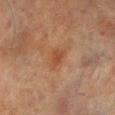Captured during whole-body skin photography for melanoma surveillance; the lesion was not biopsied. The lesion's longest dimension is about 2.5 mm. Automated tile analysis of the lesion measured a lesion area of about 3.5 mm², an outline eccentricity of about 0.7 (0 = round, 1 = elongated), and a symmetry-axis asymmetry near 0.3. It also reported an average lesion color of about L≈39 a*≈20 b*≈29 (CIELAB), a lesion–skin lightness drop of about 6, and a lesion-to-skin contrast of about 5.5 (normalized; higher = more distinct). And it measured border irregularity of about 2.5 on a 0–10 scale, a within-lesion color-variation index near 2/10, and radial color variation of about 0.5. The lesion is on the right lower leg. The patient is a male roughly 70 years of age. A region of skin cropped from a whole-body photographic capture, roughly 15 mm wide. Imaged with cross-polarized lighting.